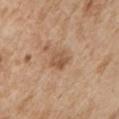biopsy status: total-body-photography surveillance lesion; no biopsy
anatomic site: the chest
patient: male, aged around 65
lighting: white-light
size: ≈3 mm
TBP lesion metrics: an outline eccentricity of about 0.6 (0 = round, 1 = elongated) and two-axis asymmetry of about 0.3; radial color variation of about 1.5
imaging modality: ~15 mm tile from a whole-body skin photo